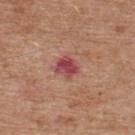lighting: white-light
diameter: ~3 mm (longest diameter)
patient: male, aged around 65
body site: the upper back
image: total-body-photography crop, ~15 mm field of view
TBP lesion metrics: a footprint of about 5.5 mm², a shape eccentricity near 0.45, and a shape-asymmetry score of about 0.25 (0 = symmetric); a lesion-detection confidence of about 100/100